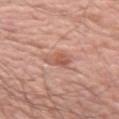<case>
  <patient>
    <sex>male</sex>
    <age_approx>50</age_approx>
  </patient>
  <site>arm</site>
  <image>
    <source>total-body photography crop</source>
    <field_of_view_mm>15</field_of_view_mm>
  </image>
</case>